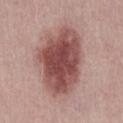Recorded during total-body skin imaging; not selected for excision or biopsy. A region of skin cropped from a whole-body photographic capture, roughly 15 mm wide. A male patient, in their 30s. Measured at roughly 9 mm in maximum diameter.